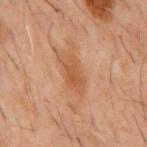workup: no biopsy performed (imaged during a skin exam); anatomic site: the mid back; tile lighting: cross-polarized illumination; patient: male, roughly 60 years of age; imaging modality: ~15 mm crop, total-body skin-cancer survey; size: about 4.5 mm.